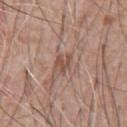{
  "biopsy_status": "not biopsied; imaged during a skin examination",
  "site": "chest",
  "image": {
    "source": "total-body photography crop",
    "field_of_view_mm": 15
  },
  "lighting": "white-light",
  "automated_metrics": {
    "vs_skin_darker_L": 9.0,
    "vs_skin_contrast_norm": 6.5
  },
  "patient": {
    "sex": "male",
    "age_approx": 60
  }
}